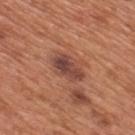Clinical impression:
Imaged during a routine full-body skin examination; the lesion was not biopsied and no histopathology is available.
Image and clinical context:
The lesion is on the mid back. A male subject, aged approximately 65. A roughly 15 mm field-of-view crop from a total-body skin photograph.